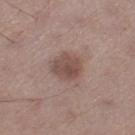* biopsy status · catalogued during a skin exam; not biopsied
* imaging modality · 15 mm crop, total-body photography
* body site · the left thigh
* subject · male, roughly 70 years of age